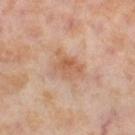Findings:
- patient · female, aged 53 to 57
- illumination · cross-polarized illumination
- image · ~15 mm crop, total-body skin-cancer survey
- body site · the right thigh